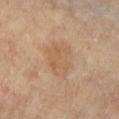Findings:
– notes: imaged on a skin check; not biopsied
– patient: female, aged 63–67
– imaging modality: ~15 mm crop, total-body skin-cancer survey
– site: the right leg
– diameter: ~4.5 mm (longest diameter)